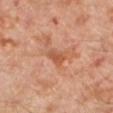biopsy status: catalogued during a skin exam; not biopsied
image source: total-body-photography crop, ~15 mm field of view
lighting: cross-polarized illumination
location: the left lower leg
subject: male, in their 30s